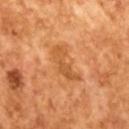Case summary:
- biopsy status: imaged on a skin check; not biopsied
- TBP lesion metrics: a shape eccentricity near 0.9 and a shape-asymmetry score of about 0.4 (0 = symmetric); a border-irregularity rating of about 5/10, a color-variation rating of about 3/10, and peripheral color asymmetry of about 1
- subject: male, aged 63 to 67
- tile lighting: cross-polarized illumination
- image source: 15 mm crop, total-body photography